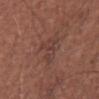workup = total-body-photography surveillance lesion; no biopsy
subject = male, aged 63 to 67
acquisition = ~15 mm tile from a whole-body skin photo
anatomic site = the abdomen